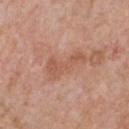Assessment:
Imaged during a routine full-body skin examination; the lesion was not biopsied and no histopathology is available.
Context:
The total-body-photography lesion software estimated a color-variation rating of about 1.5/10 and peripheral color asymmetry of about 0.5. And it measured a nevus-likeness score of about 0/100 and a lesion-detection confidence of about 100/100. Measured at roughly 5 mm in maximum diameter. The tile uses white-light illumination. A 15 mm close-up tile from a total-body photography series done for melanoma screening. On the front of the torso. A male patient, aged around 60.